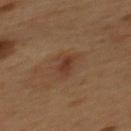| key | value |
|---|---|
| notes | catalogued during a skin exam; not biopsied |
| TBP lesion metrics | a lesion area of about 3.5 mm², an outline eccentricity of about 0.85 (0 = round, 1 = elongated), and a symmetry-axis asymmetry near 0.3; lesion-presence confidence of about 100/100 |
| acquisition | ~15 mm crop, total-body skin-cancer survey |
| tile lighting | cross-polarized illumination |
| patient | male, aged around 55 |
| size | about 2.5 mm |
| body site | the mid back |